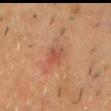  biopsy_status: not biopsied; imaged during a skin examination
  patient:
    sex: male
    age_approx: 45
  lesion_size:
    long_diameter_mm_approx: 3.0
  image:
    source: total-body photography crop
    field_of_view_mm: 15
  lighting: cross-polarized
  site: chest